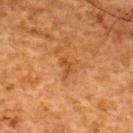Clinical impression: No biopsy was performed on this lesion — it was imaged during a full skin examination and was not determined to be concerning. Context: Located on the upper back. A 15 mm close-up tile from a total-body photography series done for melanoma screening. A male subject roughly 60 years of age. Approximately 2.5 mm at its widest. The tile uses cross-polarized illumination. Automated tile analysis of the lesion measured border irregularity of about 5 on a 0–10 scale, internal color variation of about 0 on a 0–10 scale, and a peripheral color-asymmetry measure near 0.